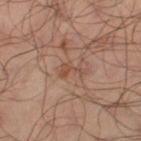Notes:
* biopsy status — imaged on a skin check; not biopsied
* anatomic site — the left thigh
* image — 15 mm crop, total-body photography
* patient — male, roughly 65 years of age
* illumination — cross-polarized
* lesion size — ~3 mm (longest diameter)